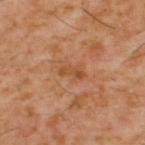Q: Was this lesion biopsied?
A: total-body-photography surveillance lesion; no biopsy
Q: Patient demographics?
A: male, about 60 years old
Q: How was this image acquired?
A: ~15 mm tile from a whole-body skin photo
Q: Automated lesion metrics?
A: a color-variation rating of about 1/10 and peripheral color asymmetry of about 0; a nevus-likeness score of about 0/100 and a detector confidence of about 100 out of 100 that the crop contains a lesion
Q: Where on the body is the lesion?
A: the upper back
Q: Lesion size?
A: about 3 mm